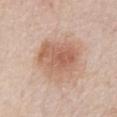Acquisition and patient details: A region of skin cropped from a whole-body photographic capture, roughly 15 mm wide. The lesion's longest dimension is about 5.5 mm. The lesion is on the abdomen. The patient is a male about 75 years old. The total-body-photography lesion software estimated a lesion color around L≈61 a*≈21 b*≈30 in CIELAB and a normalized border contrast of about 7. The software also gave a color-variation rating of about 4.5/10. Imaged with white-light lighting.